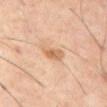Imaged during a routine full-body skin examination; the lesion was not biopsied and no histopathology is available. Cropped from a total-body skin-imaging series; the visible field is about 15 mm. The lesion-visualizer software estimated a footprint of about 5 mm², an eccentricity of roughly 0.8, and two-axis asymmetry of about 0.25. The software also gave a border-irregularity rating of about 2.5/10, a color-variation rating of about 4.5/10, and radial color variation of about 1.5. It also reported a classifier nevus-likeness of about 25/100. A male patient aged 58–62. The lesion is located on the abdomen. About 3 mm across.